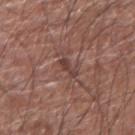The tile uses white-light illumination. Automated image analysis of the tile measured a border-irregularity rating of about 5/10 and peripheral color asymmetry of about 0. The analysis additionally found a classifier nevus-likeness of about 0/100. Located on the arm. The subject is a male roughly 75 years of age. Cropped from a total-body skin-imaging series; the visible field is about 15 mm.